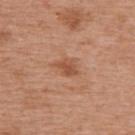{
  "biopsy_status": "not biopsied; imaged during a skin examination",
  "image": {
    "source": "total-body photography crop",
    "field_of_view_mm": 15
  },
  "patient": {
    "sex": "female",
    "age_approx": 40
  },
  "lesion_size": {
    "long_diameter_mm_approx": 3.0
  },
  "lighting": "white-light",
  "site": "upper back"
}